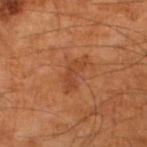The lesion was tiled from a total-body skin photograph and was not biopsied.
A roughly 15 mm field-of-view crop from a total-body skin photograph.
About 4.5 mm across.
The subject is a male in their mid-60s.
Captured under cross-polarized illumination.
Automated tile analysis of the lesion measured an area of roughly 5.5 mm², a shape eccentricity near 0.9, and a shape-asymmetry score of about 0.45 (0 = symmetric). And it measured a normalized border contrast of about 5.5. The analysis additionally found a color-variation rating of about 2/10. And it measured a nevus-likeness score of about 0/100 and a lesion-detection confidence of about 100/100.
The lesion is located on the left lower leg.